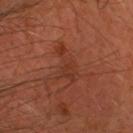Recorded during total-body skin imaging; not selected for excision or biopsy. A close-up tile cropped from a whole-body skin photograph, about 15 mm across. The recorded lesion diameter is about 4.5 mm. A male patient aged around 40. Automated tile analysis of the lesion measured a footprint of about 7.5 mm², an eccentricity of roughly 0.85, and a symmetry-axis asymmetry near 0.6. The analysis additionally found about 6 CIELAB-L* units darker than the surrounding skin. The analysis additionally found a border-irregularity rating of about 8/10 and radial color variation of about 0.5. The lesion is on the head or neck.